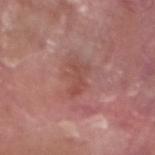Case summary:
– workup · imaged on a skin check; not biopsied
– patient · male, approximately 40 years of age
– location · the leg
– imaging modality · 15 mm crop, total-body photography